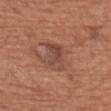| field | value |
|---|---|
| patient | female, aged around 75 |
| lesion size | ≈3.5 mm |
| acquisition | ~15 mm crop, total-body skin-cancer survey |
| tile lighting | white-light illumination |
| location | the upper back |